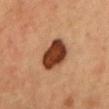Clinical impression:
This lesion was catalogued during total-body skin photography and was not selected for biopsy.
Acquisition and patient details:
Cropped from a total-body skin-imaging series; the visible field is about 15 mm. A female subject, aged 33 to 37. The tile uses cross-polarized illumination. Measured at roughly 5 mm in maximum diameter. The lesion is on the head or neck.